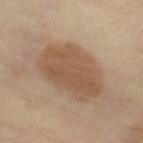The lesion was photographed on a routine skin check and not biopsied; there is no pathology result.
Cropped from a total-body skin-imaging series; the visible field is about 15 mm.
A female subject, aged 48–52.
Automated tile analysis of the lesion measured a lesion color around L≈54 a*≈18 b*≈31 in CIELAB, about 10 CIELAB-L* units darker than the surrounding skin, and a lesion-to-skin contrast of about 7.5 (normalized; higher = more distinct). The analysis additionally found a classifier nevus-likeness of about 95/100 and a lesion-detection confidence of about 100/100.
Located on the left thigh.